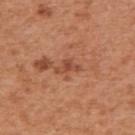A male patient, aged around 55. This is a white-light tile. Located on the right upper arm. Approximately 3 mm at its widest. A roughly 15 mm field-of-view crop from a total-body skin photograph.